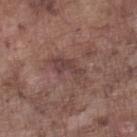Findings:
* workup: total-body-photography surveillance lesion; no biopsy
* lesion diameter: about 5 mm
* patient: male, in their mid- to late 70s
* image-analysis metrics: an area of roughly 9 mm², a shape eccentricity near 0.9, and two-axis asymmetry of about 0.35; a normalized border contrast of about 6; border irregularity of about 5.5 on a 0–10 scale, internal color variation of about 3.5 on a 0–10 scale, and radial color variation of about 1
* image source: ~15 mm tile from a whole-body skin photo
* anatomic site: the left lower leg